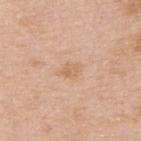Impression: Captured during whole-body skin photography for melanoma surveillance; the lesion was not biopsied. Clinical summary: The lesion's longest dimension is about 3 mm. A female patient, in their mid- to late 40s. A close-up tile cropped from a whole-body skin photograph, about 15 mm across. The lesion is on the right upper arm.